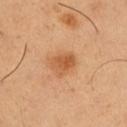{
  "biopsy_status": "not biopsied; imaged during a skin examination",
  "patient": {
    "sex": "male",
    "age_approx": 50
  },
  "site": "leg",
  "image": {
    "source": "total-body photography crop",
    "field_of_view_mm": 15
  }
}